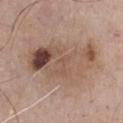Case summary:
• notes: imaged on a skin check; not biopsied
• imaging modality: ~15 mm crop, total-body skin-cancer survey
• size: ≈9.5 mm
• illumination: white-light illumination
• body site: the chest
• subject: male, aged around 70